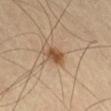Recorded during total-body skin imaging; not selected for excision or biopsy. On the right thigh. A male subject aged approximately 65. A region of skin cropped from a whole-body photographic capture, roughly 15 mm wide. Approximately 2.5 mm at its widest.